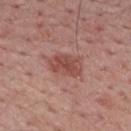No biopsy was performed on this lesion — it was imaged during a full skin examination and was not determined to be concerning. A region of skin cropped from a whole-body photographic capture, roughly 15 mm wide. The patient is a male in their mid-50s. The lesion is on the mid back.